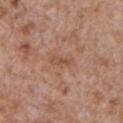Impression:
Part of a total-body skin-imaging series; this lesion was reviewed on a skin check and was not flagged for biopsy.
Acquisition and patient details:
Automated image analysis of the tile measured a footprint of about 3.5 mm² and a shape-asymmetry score of about 0.25 (0 = symmetric). It also reported a color-variation rating of about 1/10 and peripheral color asymmetry of about 0. It also reported a nevus-likeness score of about 0/100. Imaged with white-light lighting. From the front of the torso. Longest diameter approximately 3 mm. A male patient, aged around 65. Cropped from a whole-body photographic skin survey; the tile spans about 15 mm.